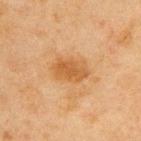Case summary:
- biopsy status · no biopsy performed (imaged during a skin exam)
- anatomic site · the upper back
- lesion size · ≈4 mm
- patient · male, in their mid-40s
- imaging modality · ~15 mm crop, total-body skin-cancer survey